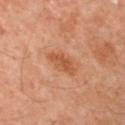notes: total-body-photography surveillance lesion; no biopsy
subject: female, aged 58 to 62
illumination: cross-polarized illumination
site: the left arm
image source: 15 mm crop, total-body photography
TBP lesion metrics: border irregularity of about 2.5 on a 0–10 scale, internal color variation of about 2 on a 0–10 scale, and peripheral color asymmetry of about 0.5